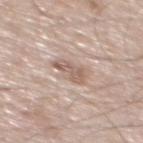Impression: Recorded during total-body skin imaging; not selected for excision or biopsy. Background: Measured at roughly 3.5 mm in maximum diameter. A roughly 15 mm field-of-view crop from a total-body skin photograph. This is a white-light tile. The lesion is located on the mid back. The subject is a male about 60 years old.